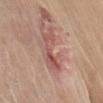– workup · imaged on a skin check; not biopsied